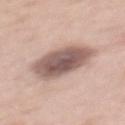No biopsy was performed on this lesion — it was imaged during a full skin examination and was not determined to be concerning.
The lesion is located on the upper back.
This image is a 15 mm lesion crop taken from a total-body photograph.
A female patient, aged 48–52.